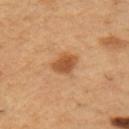Q: Is there a histopathology result?
A: total-body-photography surveillance lesion; no biopsy
Q: What are the patient's age and sex?
A: male, in their mid-50s
Q: What kind of image is this?
A: ~15 mm tile from a whole-body skin photo
Q: Illumination type?
A: cross-polarized
Q: Where on the body is the lesion?
A: the right upper arm
Q: What is the lesion's diameter?
A: ≈3 mm
Q: What did automated image analysis measure?
A: a footprint of about 6 mm², an outline eccentricity of about 0.5 (0 = round, 1 = elongated), and a shape-asymmetry score of about 0.2 (0 = symmetric); an average lesion color of about L≈51 a*≈23 b*≈38 (CIELAB) and roughly 12 lightness units darker than nearby skin; a border-irregularity index near 2/10, a color-variation rating of about 3/10, and a peripheral color-asymmetry measure near 1